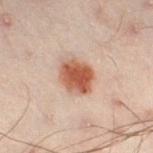Q: Was a biopsy performed?
A: imaged on a skin check; not biopsied
Q: How was this image acquired?
A: total-body-photography crop, ~15 mm field of view
Q: What did automated image analysis measure?
A: an area of roughly 11 mm², an outline eccentricity of about 0.55 (0 = round, 1 = elongated), and a symmetry-axis asymmetry near 0.15; a nevus-likeness score of about 100/100
Q: What is the anatomic site?
A: the left lower leg
Q: Who is the patient?
A: male, about 50 years old